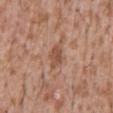biopsy status: imaged on a skin check; not biopsied
image source: ~15 mm tile from a whole-body skin photo
subject: male, roughly 45 years of age
location: the abdomen